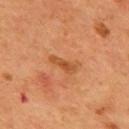{"biopsy_status": "not biopsied; imaged during a skin examination", "site": "upper back", "lesion_size": {"long_diameter_mm_approx": 3.5}, "patient": {"sex": "male", "age_approx": 55}, "image": {"source": "total-body photography crop", "field_of_view_mm": 15}, "lighting": "cross-polarized"}